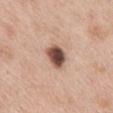Recorded during total-body skin imaging; not selected for excision or biopsy. About 3 mm across. Cropped from a whole-body photographic skin survey; the tile spans about 15 mm. An algorithmic analysis of the crop reported an eccentricity of roughly 0.6. It also reported a lesion color around L≈50 a*≈20 b*≈26 in CIELAB and about 20 CIELAB-L* units darker than the surrounding skin. The analysis additionally found a classifier nevus-likeness of about 95/100 and a detector confidence of about 100 out of 100 that the crop contains a lesion. On the mid back. A female subject, roughly 40 years of age.